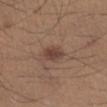Clinical impression: The lesion was tiled from a total-body skin photograph and was not biopsied. Clinical summary: This is a white-light tile. The lesion's longest dimension is about 2.5 mm. Located on the left lower leg. A roughly 15 mm field-of-view crop from a total-body skin photograph. A male patient, roughly 45 years of age.